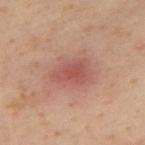Findings:
* workup · imaged on a skin check; not biopsied
* image · 15 mm crop, total-body photography
* patient · male, approximately 50 years of age
* tile lighting · cross-polarized illumination
* location · the upper back